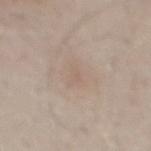The lesion was tiled from a total-body skin photograph and was not biopsied. Cropped from a whole-body photographic skin survey; the tile spans about 15 mm. Captured under white-light illumination. Located on the mid back. The subject is a male aged around 30. About 3 mm across.